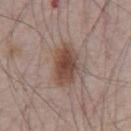This lesion was catalogued during total-body skin photography and was not selected for biopsy. A male subject, aged 63 to 67. The total-body-photography lesion software estimated a footprint of about 12 mm² and an eccentricity of roughly 0.75. The software also gave an average lesion color of about L≈46 a*≈18 b*≈25 (CIELAB) and roughly 12 lightness units darker than nearby skin. A 15 mm close-up extracted from a 3D total-body photography capture. Imaged with white-light lighting. On the chest. About 4.5 mm across.